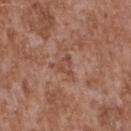{
  "biopsy_status": "not biopsied; imaged during a skin examination",
  "patient": {
    "sex": "male",
    "age_approx": 45
  },
  "image": {
    "source": "total-body photography crop",
    "field_of_view_mm": 15
  },
  "lighting": "white-light",
  "lesion_size": {
    "long_diameter_mm_approx": 2.5
  },
  "site": "upper back",
  "automated_metrics": {
    "eccentricity": 0.65,
    "shape_asymmetry": 0.5,
    "cielab_L": 48,
    "cielab_a": 22,
    "cielab_b": 28,
    "vs_skin_darker_L": 7.0,
    "vs_skin_contrast_norm": 5.5,
    "border_irregularity_0_10": 4.5,
    "color_variation_0_10": 1.5
  }
}